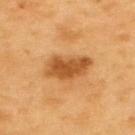The lesion was tiled from a total-body skin photograph and was not biopsied. The lesion is on the upper back. The tile uses cross-polarized illumination. A male subject, aged 58 to 62. A 15 mm close-up extracted from a 3D total-body photography capture.